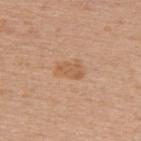<case>
<biopsy_status>not biopsied; imaged during a skin examination</biopsy_status>
<automated_metrics>
  <area_mm2_approx>5.5</area_mm2_approx>
  <eccentricity>0.8</eccentricity>
  <shape_asymmetry>0.25</shape_asymmetry>
  <border_irregularity_0_10>2.5</border_irregularity_0_10>
  <color_variation_0_10>2.0</color_variation_0_10>
  <nevus_likeness_0_100>5</nevus_likeness_0_100>
</automated_metrics>
<lighting>white-light</lighting>
<site>upper back</site>
<image>
  <source>total-body photography crop</source>
  <field_of_view_mm>15</field_of_view_mm>
</image>
<patient>
  <sex>female</sex>
  <age_approx>65</age_approx>
</patient>
</case>